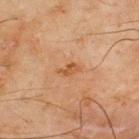Imaged during a routine full-body skin examination; the lesion was not biopsied and no histopathology is available. On the back. The subject is a male in their mid-40s. Measured at roughly 2.5 mm in maximum diameter. A 15 mm close-up extracted from a 3D total-body photography capture.